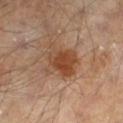<tbp_lesion>
<biopsy_status>not biopsied; imaged during a skin examination</biopsy_status>
<patient>
  <sex>male</sex>
  <age_approx>65</age_approx>
</patient>
<image>
  <source>total-body photography crop</source>
  <field_of_view_mm>15</field_of_view_mm>
</image>
<site>left lower leg</site>
</tbp_lesion>